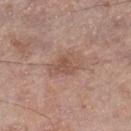Part of a total-body skin-imaging series; this lesion was reviewed on a skin check and was not flagged for biopsy.